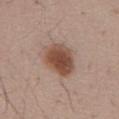workup: imaged on a skin check; not biopsied
lesion size: ~4.5 mm (longest diameter)
lighting: white-light
automated lesion analysis: a lesion area of about 13 mm² and an outline eccentricity of about 0.65 (0 = round, 1 = elongated); a nevus-likeness score of about 100/100 and a lesion-detection confidence of about 100/100
subject: male, aged approximately 60
image source: ~15 mm tile from a whole-body skin photo
site: the front of the torso